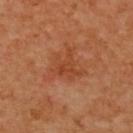Impression: No biopsy was performed on this lesion — it was imaged during a full skin examination and was not determined to be concerning. Background: Imaged with cross-polarized lighting. The lesion-visualizer software estimated roughly 6 lightness units darker than nearby skin. It also reported a nevus-likeness score of about 10/100 and a detector confidence of about 100 out of 100 that the crop contains a lesion. From the back. A 15 mm close-up extracted from a 3D total-body photography capture. A female patient, about 40 years old. Approximately 4.5 mm at its widest.